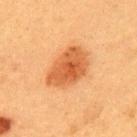biopsy_status: not biopsied; imaged during a skin examination
site: upper back
patient:
  sex: female
  age_approx: 40
image:
  source: total-body photography crop
  field_of_view_mm: 15